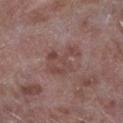Recorded during total-body skin imaging; not selected for excision or biopsy.
Measured at roughly 5 mm in maximum diameter.
The tile uses white-light illumination.
Cropped from a total-body skin-imaging series; the visible field is about 15 mm.
From the left lower leg.
A male patient about 55 years old.
The lesion-visualizer software estimated an eccentricity of roughly 0.75 and a symmetry-axis asymmetry near 0.4. The software also gave a border-irregularity rating of about 6/10, a color-variation rating of about 3.5/10, and a peripheral color-asymmetry measure near 1.5. The analysis additionally found a classifier nevus-likeness of about 0/100 and a detector confidence of about 100 out of 100 that the crop contains a lesion.